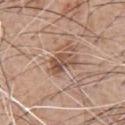This lesion was catalogued during total-body skin photography and was not selected for biopsy. A male subject aged approximately 60. A 15 mm close-up extracted from a 3D total-body photography capture. The total-body-photography lesion software estimated an area of roughly 6.5 mm², a shape eccentricity near 0.75, and two-axis asymmetry of about 0.35. It also reported a lesion color around L≈52 a*≈19 b*≈28 in CIELAB, a lesion–skin lightness drop of about 11, and a lesion-to-skin contrast of about 8 (normalized; higher = more distinct). It also reported a nevus-likeness score of about 40/100 and a detector confidence of about 100 out of 100 that the crop contains a lesion. Imaged with white-light lighting. The lesion is on the chest.